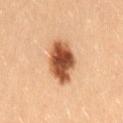biopsy status — no biopsy performed (imaged during a skin exam)
subject — female, in their mid-20s
site — the lower back
imaging modality — total-body-photography crop, ~15 mm field of view
illumination — cross-polarized